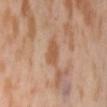Impression: No biopsy was performed on this lesion — it was imaged during a full skin examination and was not determined to be concerning. Image and clinical context: A female patient, aged 53 to 57. Approximately 2.5 mm at its widest. A 15 mm close-up extracted from a 3D total-body photography capture. The lesion is located on the lower back. Automated tile analysis of the lesion measured a footprint of about 5 mm² and a symmetry-axis asymmetry near 0.2. The software also gave a within-lesion color-variation index near 1.5/10 and radial color variation of about 0.5.